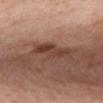Image and clinical context:
The recorded lesion diameter is about 5 mm. A region of skin cropped from a whole-body photographic capture, roughly 15 mm wide. On the chest. This is a white-light tile. The subject is a female aged 58 to 62.